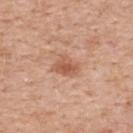Imaged during a routine full-body skin examination; the lesion was not biopsied and no histopathology is available. The patient is a female aged approximately 40. The lesion is located on the back. A 15 mm close-up tile from a total-body photography series done for melanoma screening. The lesion's longest dimension is about 3 mm. The lesion-visualizer software estimated a footprint of about 5.5 mm² and an eccentricity of roughly 0.75. And it measured an average lesion color of about L≈57 a*≈24 b*≈32 (CIELAB), a lesion–skin lightness drop of about 10, and a normalized border contrast of about 7. And it measured a border-irregularity index near 2.5/10 and a color-variation rating of about 3.5/10.